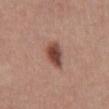| key | value |
|---|---|
| workup | imaged on a skin check; not biopsied |
| anatomic site | the front of the torso |
| patient | male, roughly 40 years of age |
| lesion size | ≈3.5 mm |
| imaging modality | total-body-photography crop, ~15 mm field of view |